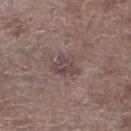Context:
The lesion is on the leg. Captured under white-light illumination. A 15 mm crop from a total-body photograph taken for skin-cancer surveillance. Approximately 4 mm at its widest. The patient is a male aged around 70.